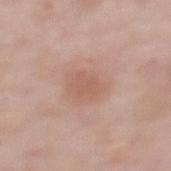• biopsy status: no biopsy performed (imaged during a skin exam)
• imaging modality: ~15 mm tile from a whole-body skin photo
• subject: female, aged 48 to 52
• site: the upper back